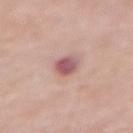The lesion was tiled from a total-body skin photograph and was not biopsied. Longest diameter approximately 3 mm. A female patient roughly 75 years of age. The lesion is located on the abdomen. A close-up tile cropped from a whole-body skin photograph, about 15 mm across. The tile uses white-light illumination.